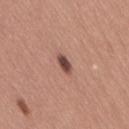Captured during whole-body skin photography for melanoma surveillance; the lesion was not biopsied.
Cropped from a whole-body photographic skin survey; the tile spans about 15 mm.
From the lower back.
The lesion's longest dimension is about 2.5 mm.
Automated image analysis of the tile measured an area of roughly 3 mm² and two-axis asymmetry of about 0.2. The software also gave a lesion color around L≈47 a*≈21 b*≈24 in CIELAB, roughly 15 lightness units darker than nearby skin, and a normalized border contrast of about 11. The analysis additionally found a border-irregularity index near 2/10, a within-lesion color-variation index near 4/10, and peripheral color asymmetry of about 1.5.
The patient is a female roughly 30 years of age.
The tile uses white-light illumination.